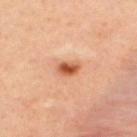Impression: The lesion was tiled from a total-body skin photograph and was not biopsied.